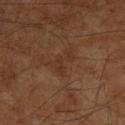{"lighting": "cross-polarized", "patient": {"sex": "male", "age_approx": 60}, "automated_metrics": {"area_mm2_approx": 5.5, "eccentricity": 0.8, "shape_asymmetry": 0.55, "cielab_L": 29, "cielab_a": 17, "cielab_b": 26, "vs_skin_darker_L": 4.0, "vs_skin_contrast_norm": 5.0, "border_irregularity_0_10": 7.5, "color_variation_0_10": 1.5, "peripheral_color_asymmetry": 0.5}, "image": {"source": "total-body photography crop", "field_of_view_mm": 15}, "site": "right lower leg"}